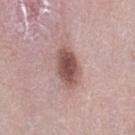Case summary:
* biopsy status: total-body-photography surveillance lesion; no biopsy
* automated lesion analysis: a symmetry-axis asymmetry near 0.15; a mean CIELAB color near L≈52 a*≈21 b*≈21, about 15 CIELAB-L* units darker than the surrounding skin, and a normalized lesion–skin contrast near 9.5; a border-irregularity rating of about 1.5/10, internal color variation of about 4.5 on a 0–10 scale, and peripheral color asymmetry of about 1.5; an automated nevus-likeness rating near 85 out of 100 and lesion-presence confidence of about 100/100
* illumination: white-light illumination
* lesion diameter: about 5 mm
* image source: total-body-photography crop, ~15 mm field of view
* anatomic site: the left thigh
* subject: female, roughly 40 years of age